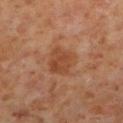Impression:
This lesion was catalogued during total-body skin photography and was not selected for biopsy.
Acquisition and patient details:
A 15 mm crop from a total-body photograph taken for skin-cancer surveillance. From the left lower leg. Approximately 4 mm at its widest. The subject is a male aged 58 to 62.